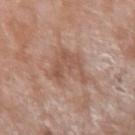Q: Is there a histopathology result?
A: no biopsy performed (imaged during a skin exam)
Q: What is the anatomic site?
A: the right forearm
Q: What did automated image analysis measure?
A: a lesion area of about 11 mm² and a shape eccentricity near 0.7
Q: Patient demographics?
A: female, aged approximately 75
Q: What is the lesion's diameter?
A: ~5 mm (longest diameter)
Q: How was this image acquired?
A: ~15 mm crop, total-body skin-cancer survey
Q: Illumination type?
A: white-light